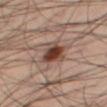- follow-up — no biopsy performed (imaged during a skin exam)
- image-analysis metrics — an area of roughly 8 mm², a shape eccentricity near 0.5, and two-axis asymmetry of about 0.25; a normalized lesion–skin contrast near 11; a border-irregularity index near 2.5/10, internal color variation of about 7.5 on a 0–10 scale, and peripheral color asymmetry of about 2
- imaging modality — ~15 mm crop, total-body skin-cancer survey
- subject — male, in their 40s
- location — the right thigh
- illumination — cross-polarized illumination